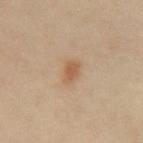{"biopsy_status": "not biopsied; imaged during a skin examination", "lighting": "cross-polarized", "lesion_size": {"long_diameter_mm_approx": 2.5}, "site": "back", "patient": {"sex": "male", "age_approx": 65}, "image": {"source": "total-body photography crop", "field_of_view_mm": 15}}